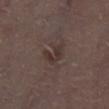Q: Was a biopsy performed?
A: catalogued during a skin exam; not biopsied
Q: What is the lesion's diameter?
A: ~3 mm (longest diameter)
Q: What lighting was used for the tile?
A: white-light illumination
Q: What kind of image is this?
A: ~15 mm crop, total-body skin-cancer survey
Q: What are the patient's age and sex?
A: male, in their 70s
Q: What is the anatomic site?
A: the left lower leg